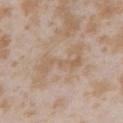Background:
Located on the left upper arm. A female patient approximately 25 years of age. Cropped from a total-body skin-imaging series; the visible field is about 15 mm.